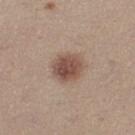Recorded during total-body skin imaging; not selected for excision or biopsy.
About 3.5 mm across.
A female subject, aged 23 to 27.
Captured under white-light illumination.
The lesion-visualizer software estimated a mean CIELAB color near L≈50 a*≈18 b*≈24, a lesion–skin lightness drop of about 13, and a normalized lesion–skin contrast near 9. The analysis additionally found a border-irregularity rating of about 1.5/10, a color-variation rating of about 4.5/10, and a peripheral color-asymmetry measure near 1.5. The software also gave an automated nevus-likeness rating near 100 out of 100 and a lesion-detection confidence of about 100/100.
A close-up tile cropped from a whole-body skin photograph, about 15 mm across.
From the left thigh.